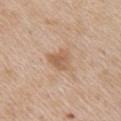Recorded during total-body skin imaging; not selected for excision or biopsy.
A close-up tile cropped from a whole-body skin photograph, about 15 mm across.
On the right upper arm.
A male patient, roughly 65 years of age.